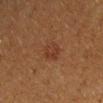Impression:
No biopsy was performed on this lesion — it was imaged during a full skin examination and was not determined to be concerning.
Clinical summary:
Imaged with cross-polarized lighting. The lesion's longest dimension is about 3 mm. The subject is a female in their 40s. Located on the arm. This image is a 15 mm lesion crop taken from a total-body photograph.